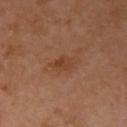Captured during whole-body skin photography for melanoma surveillance; the lesion was not biopsied. The lesion's longest dimension is about 2.5 mm. A female patient, about 55 years old. A roughly 15 mm field-of-view crop from a total-body skin photograph. The lesion is located on the arm.